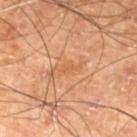{
  "biopsy_status": "not biopsied; imaged during a skin examination",
  "lesion_size": {
    "long_diameter_mm_approx": 3.0
  },
  "patient": {
    "sex": "male",
    "age_approx": 70
  },
  "automated_metrics": {
    "border_irregularity_0_10": 4.5,
    "color_variation_0_10": 0.5,
    "peripheral_color_asymmetry": 0.0
  },
  "site": "left lower leg",
  "image": {
    "source": "total-body photography crop",
    "field_of_view_mm": 15
  }
}